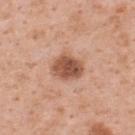– workup — no biopsy performed (imaged during a skin exam)
– patient — female, about 50 years old
– illumination — white-light
– size — ≈3.5 mm
– automated metrics — a lesion area of about 9 mm², a shape eccentricity near 0.55, and a shape-asymmetry score of about 0.15 (0 = symmetric)
– anatomic site — the upper back
– image source — total-body-photography crop, ~15 mm field of view